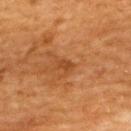This lesion was catalogued during total-body skin photography and was not selected for biopsy. From the upper back. The patient is a male aged 58–62. A region of skin cropped from a whole-body photographic capture, roughly 15 mm wide.